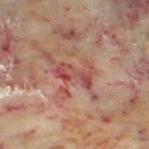<case>
<biopsy_status>not biopsied; imaged during a skin examination</biopsy_status>
<site>left thigh</site>
<image>
  <source>total-body photography crop</source>
  <field_of_view_mm>15</field_of_view_mm>
</image>
<lesion_size>
  <long_diameter_mm_approx>4.5</long_diameter_mm_approx>
</lesion_size>
<automated_metrics>
  <nevus_likeness_0_100>0</nevus_likeness_0_100>
  <lesion_detection_confidence_0_100>60</lesion_detection_confidence_0_100>
</automated_metrics>
<patient>
  <sex>female</sex>
  <age_approx>60</age_approx>
</patient>
</case>